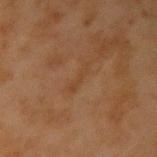Assessment: Recorded during total-body skin imaging; not selected for excision or biopsy. Acquisition and patient details: The lesion is on the left upper arm. The lesion-visualizer software estimated an average lesion color of about L≈33 a*≈17 b*≈29 (CIELAB), a lesion–skin lightness drop of about 4, and a lesion-to-skin contrast of about 4.5 (normalized; higher = more distinct). It also reported border irregularity of about 7.5 on a 0–10 scale, a within-lesion color-variation index near 0/10, and a peripheral color-asymmetry measure near 0. A male subject in their mid-40s. This is a cross-polarized tile. A 15 mm close-up extracted from a 3D total-body photography capture. The recorded lesion diameter is about 3.5 mm.